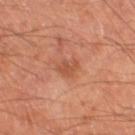follow-up — no biopsy performed (imaged during a skin exam); anatomic site — the left thigh; subject — male, in their mid- to late 60s; image source — ~15 mm crop, total-body skin-cancer survey.